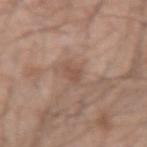Findings:
* body site · the arm
* patient · male, approximately 45 years of age
* automated metrics · an area of roughly 3 mm², a shape eccentricity near 0.7, and a shape-asymmetry score of about 0.45 (0 = symmetric); border irregularity of about 4 on a 0–10 scale, a color-variation rating of about 1/10, and radial color variation of about 0.5; lesion-presence confidence of about 100/100
* size · about 2.5 mm
* imaging modality · 15 mm crop, total-body photography